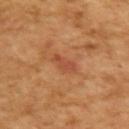Imaged during a routine full-body skin examination; the lesion was not biopsied and no histopathology is available. This is a cross-polarized tile. The lesion's longest dimension is about 3 mm. A male subject in their mid- to late 60s. A 15 mm crop from a total-body photograph taken for skin-cancer surveillance.